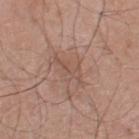<lesion>
  <image>
    <source>total-body photography crop</source>
    <field_of_view_mm>15</field_of_view_mm>
  </image>
  <site>right lower leg</site>
  <patient>
    <sex>male</sex>
    <age_approx>60</age_approx>
  </patient>
</lesion>